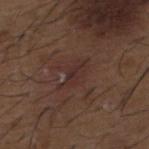Clinical impression: The lesion was tiled from a total-body skin photograph and was not biopsied. Background: The patient is a male in their 50s. From the upper back. This is a white-light tile. A roughly 15 mm field-of-view crop from a total-body skin photograph. The lesion's longest dimension is about 4 mm. An algorithmic analysis of the crop reported an eccentricity of roughly 0.9 and a symmetry-axis asymmetry near 0.4. It also reported a nevus-likeness score of about 0/100 and a lesion-detection confidence of about 90/100.